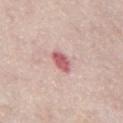This lesion was catalogued during total-body skin photography and was not selected for biopsy.
From the mid back.
A region of skin cropped from a whole-body photographic capture, roughly 15 mm wide.
A male patient aged 73 to 77.
The total-body-photography lesion software estimated an area of roughly 4.5 mm², a shape eccentricity near 0.65, and two-axis asymmetry of about 0.15. And it measured a color-variation rating of about 3/10 and a peripheral color-asymmetry measure near 1. The software also gave a lesion-detection confidence of about 100/100.
Measured at roughly 3 mm in maximum diameter.
This is a white-light tile.